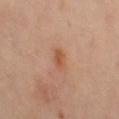The lesion was photographed on a routine skin check and not biopsied; there is no pathology result.
Cropped from a whole-body photographic skin survey; the tile spans about 15 mm.
The lesion is located on the right leg.
A female subject aged approximately 60.